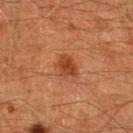Q: Was this lesion biopsied?
A: total-body-photography surveillance lesion; no biopsy
Q: Where on the body is the lesion?
A: the right lower leg
Q: Who is the patient?
A: male, aged 58–62
Q: What kind of image is this?
A: total-body-photography crop, ~15 mm field of view
Q: What is the lesion's diameter?
A: ≈3 mm
Q: What lighting was used for the tile?
A: cross-polarized